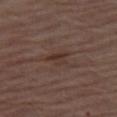Q: Was this lesion biopsied?
A: no biopsy performed (imaged during a skin exam)
Q: Patient demographics?
A: male, aged 73–77
Q: Where on the body is the lesion?
A: the right thigh
Q: Automated lesion metrics?
A: a lesion area of about 5 mm², an eccentricity of roughly 0.85, and a shape-asymmetry score of about 0.5 (0 = symmetric)
Q: What is the imaging modality?
A: total-body-photography crop, ~15 mm field of view
Q: How large is the lesion?
A: ~3.5 mm (longest diameter)